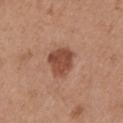Impression: Part of a total-body skin-imaging series; this lesion was reviewed on a skin check and was not flagged for biopsy. Image and clinical context: The lesion's longest dimension is about 3.5 mm. Imaged with white-light lighting. From the chest. The patient is a female aged approximately 65. A 15 mm close-up extracted from a 3D total-body photography capture. Automated tile analysis of the lesion measured lesion-presence confidence of about 100/100.